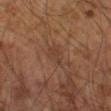Recorded during total-body skin imaging; not selected for excision or biopsy.
The lesion's longest dimension is about 3 mm.
On the left arm.
A male patient, in their mid- to late 60s.
The tile uses cross-polarized illumination.
A region of skin cropped from a whole-body photographic capture, roughly 15 mm wide.